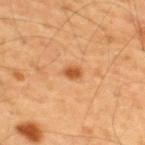This lesion was catalogued during total-body skin photography and was not selected for biopsy. The recorded lesion diameter is about 2 mm. On the upper back. Imaged with cross-polarized lighting. A close-up tile cropped from a whole-body skin photograph, about 15 mm across. A male patient, in their mid-50s.